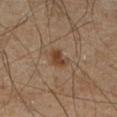Findings:
- biopsy status · imaged on a skin check; not biopsied
- subject · male, approximately 45 years of age
- location · the leg
- image source · 15 mm crop, total-body photography